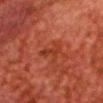Impression: No biopsy was performed on this lesion — it was imaged during a full skin examination and was not determined to be concerning. Context: The tile uses cross-polarized illumination. Measured at roughly 3.5 mm in maximum diameter. Automated tile analysis of the lesion measured a border-irregularity rating of about 5.5/10 and internal color variation of about 0.5 on a 0–10 scale. The analysis additionally found a classifier nevus-likeness of about 0/100 and a lesion-detection confidence of about 100/100. The lesion is on the head or neck. A male subject, in their 60s. A close-up tile cropped from a whole-body skin photograph, about 15 mm across.